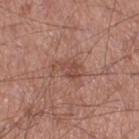The lesion was tiled from a total-body skin photograph and was not biopsied. A lesion tile, about 15 mm wide, cut from a 3D total-body photograph. The lesion is located on the right thigh. The total-body-photography lesion software estimated a classifier nevus-likeness of about 5/100. Approximately 3 mm at its widest. A male patient, aged 53 to 57. Imaged with white-light lighting.